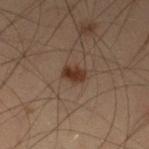Q: Is there a histopathology result?
A: total-body-photography surveillance lesion; no biopsy
Q: Patient demographics?
A: male, in their mid- to late 50s
Q: What kind of image is this?
A: ~15 mm tile from a whole-body skin photo
Q: Lesion location?
A: the left lower leg
Q: What lighting was used for the tile?
A: cross-polarized illumination